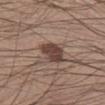follow-up: catalogued during a skin exam; not biopsied | subject: male, roughly 30 years of age | lesion size: ~3.5 mm (longest diameter) | body site: the left lower leg | tile lighting: white-light illumination | automated metrics: a footprint of about 8 mm² and a shape-asymmetry score of about 0.15 (0 = symmetric); internal color variation of about 3 on a 0–10 scale and peripheral color asymmetry of about 1 | imaging modality: 15 mm crop, total-body photography.